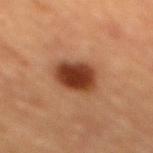The lesion was tiled from a total-body skin photograph and was not biopsied.
Approximately 4 mm at its widest.
The tile uses cross-polarized illumination.
From the mid back.
A close-up tile cropped from a whole-body skin photograph, about 15 mm across.
The patient is a female roughly 70 years of age.